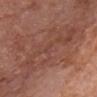{"image": {"source": "total-body photography crop", "field_of_view_mm": 15}, "patient": {"sex": "male", "age_approx": 65}, "site": "chest", "lesion_size": {"long_diameter_mm_approx": 9.0}, "lighting": "white-light"}